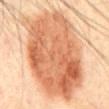Case summary:
• follow-up — imaged on a skin check; not biopsied
• diameter — ≈12 mm
• location — the abdomen
• automated lesion analysis — an area of roughly 75 mm²; a lesion color around L≈62 a*≈24 b*≈37 in CIELAB, about 14 CIELAB-L* units darker than the surrounding skin, and a normalized lesion–skin contrast near 9; a border-irregularity rating of about 2/10, internal color variation of about 7.5 on a 0–10 scale, and peripheral color asymmetry of about 2.5; a lesion-detection confidence of about 100/100
• patient — male, roughly 45 years of age
• illumination — cross-polarized
• image — total-body-photography crop, ~15 mm field of view